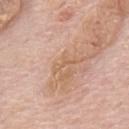This lesion was catalogued during total-body skin photography and was not selected for biopsy.
The recorded lesion diameter is about 3 mm.
Captured under white-light illumination.
A male patient, aged approximately 75.
A region of skin cropped from a whole-body photographic capture, roughly 15 mm wide.
The lesion is on the upper back.
The total-body-photography lesion software estimated a shape-asymmetry score of about 0.75 (0 = symmetric). And it measured a mean CIELAB color near L≈61 a*≈19 b*≈34 and a lesion–skin lightness drop of about 6. And it measured a border-irregularity index near 8/10, internal color variation of about 0 on a 0–10 scale, and a peripheral color-asymmetry measure near 0. The software also gave a nevus-likeness score of about 0/100 and a detector confidence of about 80 out of 100 that the crop contains a lesion.